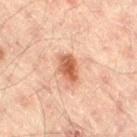Assessment:
This lesion was catalogued during total-body skin photography and was not selected for biopsy.
Background:
Measured at roughly 3.5 mm in maximum diameter. Imaged with cross-polarized lighting. The patient is a male roughly 45 years of age. This image is a 15 mm lesion crop taken from a total-body photograph. The lesion is on the leg.